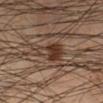follow-up — no biopsy performed (imaged during a skin exam); location — the right lower leg; lesion diameter — about 4.5 mm; acquisition — ~15 mm tile from a whole-body skin photo; patient — male, roughly 50 years of age; lighting — cross-polarized.